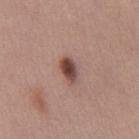biopsy_status: not biopsied; imaged during a skin examination
image:
  source: total-body photography crop
  field_of_view_mm: 15
patient:
  sex: male
  age_approx: 35
site: abdomen